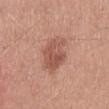Notes:
- workup — total-body-photography surveillance lesion; no biopsy
- patient — male, roughly 30 years of age
- site — the mid back
- lesion diameter — about 5.5 mm
- acquisition — 15 mm crop, total-body photography